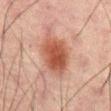{"biopsy_status": "not biopsied; imaged during a skin examination", "image": {"source": "total-body photography crop", "field_of_view_mm": 15}, "patient": {"sex": "male", "age_approx": 65}, "automated_metrics": {"cielab_L": 42, "cielab_a": 22, "cielab_b": 27, "vs_skin_darker_L": 11.0, "vs_skin_contrast_norm": 9.0, "nevus_likeness_0_100": 100}, "site": "mid back", "lesion_size": {"long_diameter_mm_approx": 5.0}}